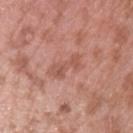lesion_size:
  long_diameter_mm_approx: 4.5
patient:
  sex: male
  age_approx: 40
image:
  source: total-body photography crop
  field_of_view_mm: 15
site: left upper arm
lighting: white-light